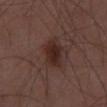– patient — male, aged 48–52
– image source — ~15 mm crop, total-body skin-cancer survey